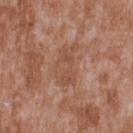| key | value |
|---|---|
| biopsy status | imaged on a skin check; not biopsied |
| patient | male, aged 43–47 |
| lighting | white-light illumination |
| lesion diameter | about 5.5 mm |
| imaging modality | total-body-photography crop, ~15 mm field of view |
| site | the upper back |